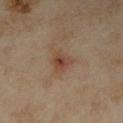follow-up: total-body-photography surveillance lesion; no biopsy
diameter: ~2.5 mm (longest diameter)
image: total-body-photography crop, ~15 mm field of view
patient: female, about 35 years old
site: the right forearm